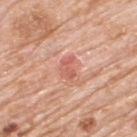Assessment:
Recorded during total-body skin imaging; not selected for excision or biopsy.
Acquisition and patient details:
A male subject about 80 years old. This is a white-light tile. A 15 mm close-up tile from a total-body photography series done for melanoma screening. The lesion is on the upper back. Approximately 2.5 mm at its widest.